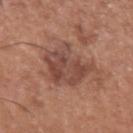– biopsy status · no biopsy performed (imaged during a skin exam)
– anatomic site · the left upper arm
– patient · male, aged 63 to 67
– tile lighting · white-light
– imaging modality · ~15 mm tile from a whole-body skin photo
– lesion diameter · about 6.5 mm
– automated metrics · a footprint of about 18 mm², an outline eccentricity of about 0.7 (0 = round, 1 = elongated), and a shape-asymmetry score of about 0.4 (0 = symmetric); a color-variation rating of about 4.5/10 and radial color variation of about 1.5